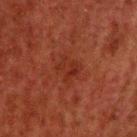anatomic site=the upper back | patient=male, about 60 years old | acquisition=~15 mm crop, total-body skin-cancer survey | lighting=cross-polarized illumination | lesion diameter=about 3 mm.